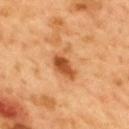The lesion was tiled from a total-body skin photograph and was not biopsied.
This image is a 15 mm lesion crop taken from a total-body photograph.
The subject is a male roughly 50 years of age.
The lesion is located on the mid back.
The lesion's longest dimension is about 4 mm.
Captured under cross-polarized illumination.
The lesion-visualizer software estimated a border-irregularity rating of about 4.5/10.